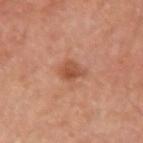Q: Was this lesion biopsied?
A: imaged on a skin check; not biopsied
Q: What lighting was used for the tile?
A: cross-polarized
Q: Lesion location?
A: the left upper arm
Q: What kind of image is this?
A: ~15 mm crop, total-body skin-cancer survey
Q: What is the lesion's diameter?
A: about 3 mm
Q: Who is the patient?
A: male, approximately 55 years of age